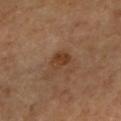Context:
Longest diameter approximately 2.5 mm. The lesion is located on the left arm. The patient is a female roughly 60 years of age. Imaged with cross-polarized lighting. A roughly 15 mm field-of-view crop from a total-body skin photograph.